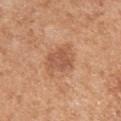{
  "biopsy_status": "not biopsied; imaged during a skin examination",
  "image": {
    "source": "total-body photography crop",
    "field_of_view_mm": 15
  },
  "lesion_size": {
    "long_diameter_mm_approx": 4.5
  },
  "patient": {
    "sex": "female",
    "age_approx": 55
  },
  "site": "left upper arm"
}